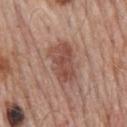Q: Lesion location?
A: the mid back
Q: Illumination type?
A: white-light illumination
Q: What kind of image is this?
A: ~15 mm crop, total-body skin-cancer survey
Q: What are the patient's age and sex?
A: male, aged approximately 75
Q: Lesion size?
A: about 5.5 mm